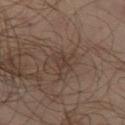notes=imaged on a skin check; not biopsied
image=~15 mm tile from a whole-body skin photo
subject=male, aged 63–67
location=the leg
diameter=~3 mm (longest diameter)